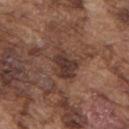Findings:
* notes — catalogued during a skin exam; not biopsied
* imaging modality — ~15 mm crop, total-body skin-cancer survey
* automated lesion analysis — a footprint of about 9.5 mm², an outline eccentricity of about 0.7 (0 = round, 1 = elongated), and two-axis asymmetry of about 0.35; an average lesion color of about L≈36 a*≈18 b*≈23 (CIELAB) and a normalized lesion–skin contrast near 8.5; a detector confidence of about 100 out of 100 that the crop contains a lesion
* tile lighting — white-light
* patient — male, aged around 75
* location — the upper back
* diameter — ≈4 mm